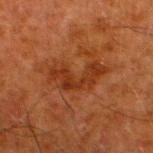Impression: Part of a total-body skin-imaging series; this lesion was reviewed on a skin check and was not flagged for biopsy. Acquisition and patient details: This image is a 15 mm lesion crop taken from a total-body photograph. From the left lower leg. This is a cross-polarized tile. Approximately 6 mm at its widest. An algorithmic analysis of the crop reported an average lesion color of about L≈28 a*≈24 b*≈31 (CIELAB), a lesion–skin lightness drop of about 7, and a normalized lesion–skin contrast near 7. And it measured border irregularity of about 7 on a 0–10 scale and a color-variation rating of about 2.5/10. A male subject, roughly 80 years of age.